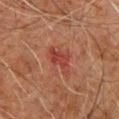The lesion was tiled from a total-body skin photograph and was not biopsied.
A close-up tile cropped from a whole-body skin photograph, about 15 mm across.
On the chest.
A male subject roughly 60 years of age.